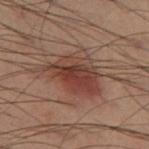{
  "image": {
    "source": "total-body photography crop",
    "field_of_view_mm": 15
  },
  "patient": {
    "sex": "male",
    "age_approx": 55
  },
  "lighting": "cross-polarized",
  "lesion_size": {
    "long_diameter_mm_approx": 6.5
  },
  "site": "left thigh"
}